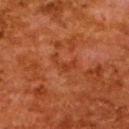<case>
  <lesion_size>
    <long_diameter_mm_approx>3.5</long_diameter_mm_approx>
  </lesion_size>
  <patient>
    <sex>female</sex>
    <age_approx>50</age_approx>
  </patient>
  <image>
    <source>total-body photography crop</source>
    <field_of_view_mm>15</field_of_view_mm>
  </image>
  <lighting>cross-polarized</lighting>
  <site>upper back</site>
  <automated_metrics>
    <area_mm2_approx>3.5</area_mm2_approx>
    <eccentricity>0.9</eccentricity>
    <cielab_L>33</cielab_L>
    <cielab_a>27</cielab_a>
    <cielab_b>33</cielab_b>
    <vs_skin_darker_L>5.0</vs_skin_darker_L>
    <vs_skin_contrast_norm>5.0</vs_skin_contrast_norm>
    <border_irregularity_0_10>7.5</border_irregularity_0_10>
    <color_variation_0_10>0.0</color_variation_0_10>
    <peripheral_color_asymmetry>0.0</peripheral_color_asymmetry>
  </automated_metrics>
</case>